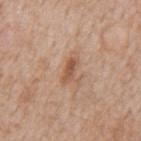Notes:
* location · the mid back
* subject · male, aged approximately 65
* image · 15 mm crop, total-body photography
* illumination · white-light illumination
* TBP lesion metrics · a border-irregularity index near 2.5/10, a within-lesion color-variation index near 3/10, and peripheral color asymmetry of about 1